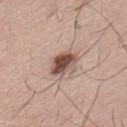Q: Is there a histopathology result?
A: total-body-photography surveillance lesion; no biopsy
Q: What lighting was used for the tile?
A: white-light
Q: Automated lesion metrics?
A: a footprint of about 7.5 mm², a shape eccentricity near 0.65, and a symmetry-axis asymmetry near 0.2; a classifier nevus-likeness of about 85/100 and lesion-presence confidence of about 100/100
Q: Where on the body is the lesion?
A: the back
Q: How large is the lesion?
A: about 3.5 mm
Q: What are the patient's age and sex?
A: male, aged around 65
Q: How was this image acquired?
A: ~15 mm crop, total-body skin-cancer survey